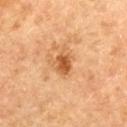Recorded during total-body skin imaging; not selected for excision or biopsy.
Approximately 3.5 mm at its widest.
Automated image analysis of the tile measured an area of roughly 6 mm², an outline eccentricity of about 0.7 (0 = round, 1 = elongated), and a shape-asymmetry score of about 0.3 (0 = symmetric). And it measured a lesion color around L≈54 a*≈24 b*≈40 in CIELAB, roughly 11 lightness units darker than nearby skin, and a normalized lesion–skin contrast near 8.
This is a cross-polarized tile.
Located on the upper back.
A 15 mm close-up extracted from a 3D total-body photography capture.
The patient is a female in their 60s.